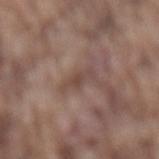  biopsy_status: not biopsied; imaged during a skin examination
  patient:
    sex: male
    age_approx: 75
  lesion_size:
    long_diameter_mm_approx: 3.5
  site: lower back
  image:
    source: total-body photography crop
    field_of_view_mm: 15
  lighting: white-light